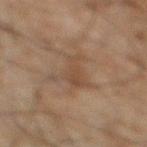Q: Was this lesion biopsied?
A: catalogued during a skin exam; not biopsied
Q: Who is the patient?
A: male, about 45 years old
Q: Illumination type?
A: cross-polarized illumination
Q: Where on the body is the lesion?
A: the right lower leg
Q: How was this image acquired?
A: ~15 mm crop, total-body skin-cancer survey
Q: What is the lesion's diameter?
A: ≈6 mm
Q: What did automated image analysis measure?
A: an area of roughly 9 mm² and a symmetry-axis asymmetry near 0.65; a color-variation rating of about 2/10 and a peripheral color-asymmetry measure near 0.5